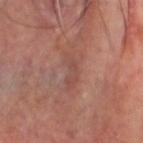Impression: The lesion was tiled from a total-body skin photograph and was not biopsied. Background: A roughly 15 mm field-of-view crop from a total-body skin photograph. Captured under cross-polarized illumination. Automated tile analysis of the lesion measured roughly 6 lightness units darker than nearby skin and a lesion-to-skin contrast of about 5 (normalized; higher = more distinct). The analysis additionally found an automated nevus-likeness rating near 0 out of 100 and lesion-presence confidence of about 65/100. The lesion is on the head or neck. A male patient aged 58 to 62. Approximately 4.5 mm at its widest.